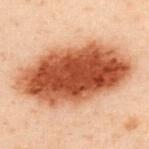A 15 mm crop from a total-body photograph taken for skin-cancer surveillance.
Imaged with cross-polarized lighting.
The lesion is on the upper back.
Automated tile analysis of the lesion measured a border-irregularity rating of about 2/10 and a peripheral color-asymmetry measure near 3. It also reported a classifier nevus-likeness of about 100/100.
About 12 mm across.
A male patient, roughly 50 years of age.